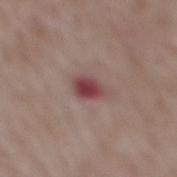biopsy_status: not biopsied; imaged during a skin examination
automated_metrics:
  color_variation_0_10: 4.5
  peripheral_color_asymmetry: 1.5
image:
  source: total-body photography crop
  field_of_view_mm: 15
patient:
  sex: female
  age_approx: 70
site: upper back
lesion_size:
  long_diameter_mm_approx: 3.0